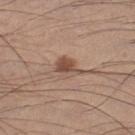{
  "biopsy_status": "not biopsied; imaged during a skin examination",
  "site": "left lower leg",
  "lesion_size": {
    "long_diameter_mm_approx": 3.0
  },
  "image": {
    "source": "total-body photography crop",
    "field_of_view_mm": 15
  },
  "patient": {
    "sex": "male",
    "age_approx": 35
  }
}